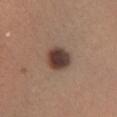Imaged during a routine full-body skin examination; the lesion was not biopsied and no histopathology is available. A female patient aged approximately 55. A 15 mm crop from a total-body photograph taken for skin-cancer surveillance. Located on the right lower leg.